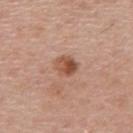This lesion was catalogued during total-body skin photography and was not selected for biopsy.
The total-body-photography lesion software estimated an outline eccentricity of about 0.45 (0 = round, 1 = elongated). The analysis additionally found a lesion color around L≈52 a*≈23 b*≈31 in CIELAB and about 11 CIELAB-L* units darker than the surrounding skin. The analysis additionally found a border-irregularity index near 2/10, a within-lesion color-variation index near 7/10, and peripheral color asymmetry of about 3. The software also gave a classifier nevus-likeness of about 85/100 and a lesion-detection confidence of about 100/100.
From the back.
A 15 mm crop from a total-body photograph taken for skin-cancer surveillance.
Approximately 3 mm at its widest.
A male subject, approximately 60 years of age.
This is a white-light tile.